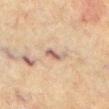This lesion was catalogued during total-body skin photography and was not selected for biopsy.
Cropped from a total-body skin-imaging series; the visible field is about 15 mm.
On the abdomen.
The patient is a male aged 73–77.
The lesion-visualizer software estimated an area of roughly 3 mm², a shape eccentricity near 0.9, and a symmetry-axis asymmetry near 0.4. It also reported a mean CIELAB color near L≈51 a*≈16 b*≈22. The software also gave peripheral color asymmetry of about 0. It also reported a nevus-likeness score of about 0/100 and a lesion-detection confidence of about 90/100.
The lesion's longest dimension is about 3 mm.
Imaged with cross-polarized lighting.